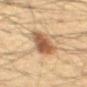notes = catalogued during a skin exam; not biopsied
image = 15 mm crop, total-body photography
body site = the abdomen
subject = male, roughly 60 years of age
lesion size = ≈5 mm